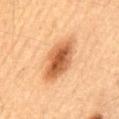Findings:
– workup · total-body-photography surveillance lesion; no biopsy
– lesion size · about 6.5 mm
– subject · male, approximately 65 years of age
– TBP lesion metrics · a lesion area of about 15 mm² and an eccentricity of roughly 0.85; a border-irregularity rating of about 2/10 and radial color variation of about 2; a nevus-likeness score of about 100/100
– anatomic site · the abdomen
– imaging modality · 15 mm crop, total-body photography
– illumination · cross-polarized